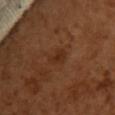Q: Was this lesion biopsied?
A: total-body-photography surveillance lesion; no biopsy
Q: What is the lesion's diameter?
A: ≈2.5 mm
Q: What kind of image is this?
A: total-body-photography crop, ~15 mm field of view
Q: How was the tile lit?
A: cross-polarized
Q: Automated lesion metrics?
A: a lesion area of about 3.5 mm² and a symmetry-axis asymmetry near 0.3; a lesion color around L≈29 a*≈22 b*≈30 in CIELAB, roughly 6 lightness units darker than nearby skin, and a normalized border contrast of about 6.5; a classifier nevus-likeness of about 20/100 and lesion-presence confidence of about 100/100
Q: Lesion location?
A: the chest
Q: Who is the patient?
A: female, roughly 55 years of age